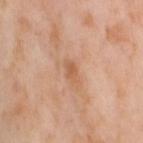No biopsy was performed on this lesion — it was imaged during a full skin examination and was not determined to be concerning. The patient is a female aged around 55. A region of skin cropped from a whole-body photographic capture, roughly 15 mm wide. On the right thigh.